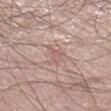The lesion was tiled from a total-body skin photograph and was not biopsied.
This image is a 15 mm lesion crop taken from a total-body photograph.
Located on the right lower leg.
A male patient, aged around 50.
Measured at roughly 3 mm in maximum diameter.
This is a white-light tile.